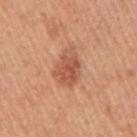Q: Who is the patient?
A: male, aged 48 to 52
Q: How was this image acquired?
A: ~15 mm tile from a whole-body skin photo
Q: How was the tile lit?
A: white-light
Q: What did automated image analysis measure?
A: a lesion area of about 9.5 mm², an eccentricity of roughly 0.7, and two-axis asymmetry of about 0.25; a mean CIELAB color near L≈55 a*≈26 b*≈33 and a lesion–skin lightness drop of about 10
Q: Lesion location?
A: the right upper arm
Q: How large is the lesion?
A: ~4 mm (longest diameter)